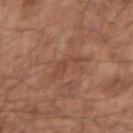The lesion was photographed on a routine skin check and not biopsied; there is no pathology result. On the right upper arm. A male patient, in their mid-50s. Approximately 4 mm at its widest. A region of skin cropped from a whole-body photographic capture, roughly 15 mm wide.